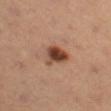workup = imaged on a skin check; not biopsied